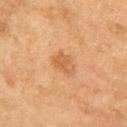Imaged during a routine full-body skin examination; the lesion was not biopsied and no histopathology is available. On the right upper arm. This image is a 15 mm lesion crop taken from a total-body photograph. About 3.5 mm across. The total-body-photography lesion software estimated roughly 8 lightness units darker than nearby skin and a lesion-to-skin contrast of about 6 (normalized; higher = more distinct). The subject is a female aged 58 to 62. This is a cross-polarized tile.